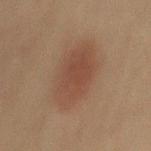Assessment: Recorded during total-body skin imaging; not selected for excision or biopsy. Acquisition and patient details: On the mid back. A male subject, about 60 years old. The tile uses cross-polarized illumination. Cropped from a total-body skin-imaging series; the visible field is about 15 mm.